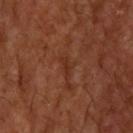Impression: Part of a total-body skin-imaging series; this lesion was reviewed on a skin check and was not flagged for biopsy. Acquisition and patient details: A 15 mm crop from a total-body photograph taken for skin-cancer surveillance. A male subject aged 53–57. The lesion is located on the back. Measured at roughly 3 mm in maximum diameter.